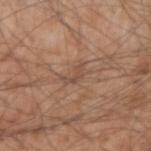Assessment:
No biopsy was performed on this lesion — it was imaged during a full skin examination and was not determined to be concerning.
Context:
Cropped from a total-body skin-imaging series; the visible field is about 15 mm. Longest diameter approximately 3 mm. An algorithmic analysis of the crop reported a shape eccentricity near 0.85 and a shape-asymmetry score of about 0.5 (0 = symmetric). The software also gave an average lesion color of about L≈49 a*≈19 b*≈28 (CIELAB), a lesion–skin lightness drop of about 7, and a normalized lesion–skin contrast near 5.5. And it measured an automated nevus-likeness rating near 0 out of 100 and lesion-presence confidence of about 55/100. The subject is a male about 55 years old. The lesion is on the right forearm.